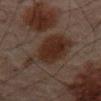follow-up: no biopsy performed (imaged during a skin exam) | patient: male, aged around 65 | image: ~15 mm crop, total-body skin-cancer survey | location: the lower back.